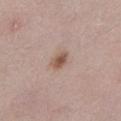notes = imaged on a skin check; not biopsied